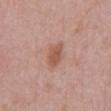Captured during whole-body skin photography for melanoma surveillance; the lesion was not biopsied. Automated image analysis of the tile measured a nevus-likeness score of about 40/100. A roughly 15 mm field-of-view crop from a total-body skin photograph. On the abdomen. A male subject aged 48–52.